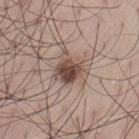biopsy status: catalogued during a skin exam; not biopsied
imaging modality: total-body-photography crop, ~15 mm field of view
lesion diameter: about 4 mm
location: the left thigh
illumination: white-light
patient: male, aged 53 to 57